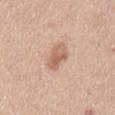From the front of the torso. The recorded lesion diameter is about 4 mm. Captured under white-light illumination. The subject is a female in their mid-50s. Cropped from a whole-body photographic skin survey; the tile spans about 15 mm.